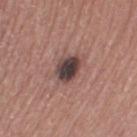Imaged during a routine full-body skin examination; the lesion was not biopsied and no histopathology is available.
The subject is a male in their mid- to late 60s.
An algorithmic analysis of the crop reported a border-irregularity rating of about 1.5/10 and a within-lesion color-variation index near 5.5/10. The analysis additionally found a classifier nevus-likeness of about 50/100 and a detector confidence of about 100 out of 100 that the crop contains a lesion.
This image is a 15 mm lesion crop taken from a total-body photograph.
Approximately 3.5 mm at its widest.
The tile uses white-light illumination.
The lesion is on the left thigh.